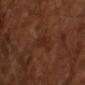Q: Was a biopsy performed?
A: total-body-photography surveillance lesion; no biopsy